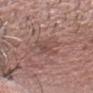follow-up — imaged on a skin check; not biopsied | imaging modality — 15 mm crop, total-body photography | automated lesion analysis — an eccentricity of roughly 0.65 and a shape-asymmetry score of about 0.35 (0 = symmetric); an average lesion color of about L≈48 a*≈19 b*≈23 (CIELAB), a lesion–skin lightness drop of about 7, and a lesion-to-skin contrast of about 5 (normalized; higher = more distinct); border irregularity of about 3.5 on a 0–10 scale, a within-lesion color-variation index near 3.5/10, and peripheral color asymmetry of about 1.5 | lesion size — about 3.5 mm | lighting — white-light | patient — male, aged around 65 | anatomic site — the head or neck.